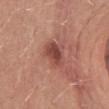Imaged during a routine full-body skin examination; the lesion was not biopsied and no histopathology is available. On the abdomen. A female subject in their mid-20s. Imaged with white-light lighting. A 15 mm close-up extracted from a 3D total-body photography capture.